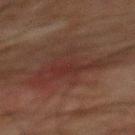Clinical impression:
Recorded during total-body skin imaging; not selected for excision or biopsy.
Context:
The tile uses cross-polarized illumination. A male patient, aged 63–67. On the back. A 15 mm close-up extracted from a 3D total-body photography capture. Automated tile analysis of the lesion measured a lesion area of about 16 mm², a shape eccentricity near 0.85, and two-axis asymmetry of about 0.6. And it measured a mean CIELAB color near L≈25 a*≈18 b*≈19, a lesion–skin lightness drop of about 5, and a normalized border contrast of about 6. Measured at roughly 7.5 mm in maximum diameter.